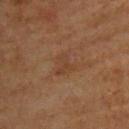Findings:
– site: the upper back
– patient: male, approximately 60 years of age
– imaging modality: ~15 mm crop, total-body skin-cancer survey